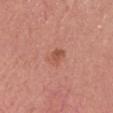Impression:
The lesion was tiled from a total-body skin photograph and was not biopsied.
Acquisition and patient details:
The total-body-photography lesion software estimated a symmetry-axis asymmetry near 0.3. The analysis additionally found roughly 8 lightness units darker than nearby skin and a lesion-to-skin contrast of about 6.5 (normalized; higher = more distinct). And it measured a within-lesion color-variation index near 2/10. Imaged with white-light lighting. A male patient, approximately 60 years of age. The lesion is on the head or neck. A 15 mm close-up tile from a total-body photography series done for melanoma screening.